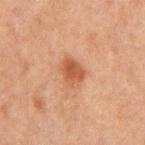The lesion was tiled from a total-body skin photograph and was not biopsied.
The recorded lesion diameter is about 3 mm.
The lesion is located on the mid back.
A 15 mm crop from a total-body photograph taken for skin-cancer surveillance.
A male subject, in their mid- to late 60s.
Automated tile analysis of the lesion measured a border-irregularity index near 1.5/10, a within-lesion color-variation index near 3.5/10, and a peripheral color-asymmetry measure near 1.